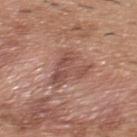Imaged during a routine full-body skin examination; the lesion was not biopsied and no histopathology is available. Captured under white-light illumination. Measured at roughly 4.5 mm in maximum diameter. An algorithmic analysis of the crop reported a mean CIELAB color near L≈50 a*≈22 b*≈25, a lesion–skin lightness drop of about 9, and a normalized lesion–skin contrast near 7. It also reported a border-irregularity index near 7.5/10, a color-variation rating of about 3.5/10, and a peripheral color-asymmetry measure near 1. The subject is a male in their 40s. Cropped from a whole-body photographic skin survey; the tile spans about 15 mm. The lesion is located on the upper back.